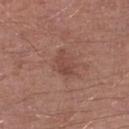The lesion was tiled from a total-body skin photograph and was not biopsied.
A 15 mm crop from a total-body photograph taken for skin-cancer surveillance.
The recorded lesion diameter is about 3 mm.
A male patient, roughly 70 years of age.
This is a white-light tile.
The lesion is on the right lower leg.